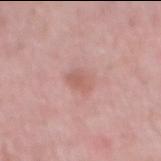| feature | finding |
|---|---|
| TBP lesion metrics | a within-lesion color-variation index near 2.5/10 and a peripheral color-asymmetry measure near 1 |
| lesion diameter | about 2.5 mm |
| acquisition | ~15 mm tile from a whole-body skin photo |
| location | the abdomen |
| subject | male, approximately 50 years of age |